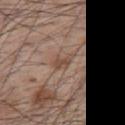biopsy_status: not biopsied; imaged during a skin examination
image:
  source: total-body photography crop
  field_of_view_mm: 15
patient:
  sex: male
  age_approx: 65
site: upper back